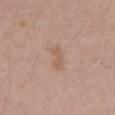{"biopsy_status": "not biopsied; imaged during a skin examination", "patient": {"sex": "male", "age_approx": 40}, "site": "arm", "image": {"source": "total-body photography crop", "field_of_view_mm": 15}, "lesion_size": {"long_diameter_mm_approx": 3.0}, "automated_metrics": {"area_mm2_approx": 4.0, "shape_asymmetry": 0.3, "cielab_L": 58, "cielab_a": 18, "cielab_b": 29, "vs_skin_darker_L": 6.0, "vs_skin_contrast_norm": 5.0, "color_variation_0_10": 1.0, "peripheral_color_asymmetry": 0.5}}